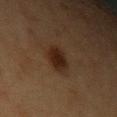Part of a total-body skin-imaging series; this lesion was reviewed on a skin check and was not flagged for biopsy. A 15 mm crop from a total-body photograph taken for skin-cancer surveillance. Captured under cross-polarized illumination. About 3.5 mm across. Located on the left upper arm. Automated tile analysis of the lesion measured a footprint of about 7 mm² and a symmetry-axis asymmetry near 0.15. And it measured a lesion-to-skin contrast of about 10 (normalized; higher = more distinct). The analysis additionally found border irregularity of about 1.5 on a 0–10 scale and radial color variation of about 1. And it measured a classifier nevus-likeness of about 95/100 and a lesion-detection confidence of about 100/100. A female patient, aged approximately 40.